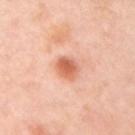follow-up = total-body-photography surveillance lesion; no biopsy | image = 15 mm crop, total-body photography | body site = the left arm | patient = female, roughly 40 years of age.